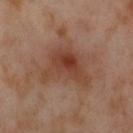biopsy status = no biopsy performed (imaged during a skin exam)